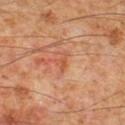Q: Is there a histopathology result?
A: total-body-photography surveillance lesion; no biopsy
Q: Who is the patient?
A: male, aged around 60
Q: Lesion location?
A: the leg
Q: What is the imaging modality?
A: total-body-photography crop, ~15 mm field of view